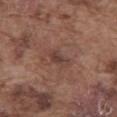<tbp_lesion>
  <biopsy_status>not biopsied; imaged during a skin examination</biopsy_status>
  <image>
    <source>total-body photography crop</source>
    <field_of_view_mm>15</field_of_view_mm>
  </image>
  <site>abdomen</site>
  <lighting>white-light</lighting>
  <lesion_size>
    <long_diameter_mm_approx>3.0</long_diameter_mm_approx>
  </lesion_size>
  <patient>
    <sex>male</sex>
    <age_approx>75</age_approx>
  </patient>
</tbp_lesion>